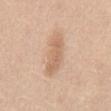Image and clinical context:
On the abdomen. This image is a 15 mm lesion crop taken from a total-body photograph. The patient is a male about 60 years old.